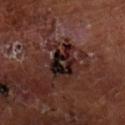The recorded lesion diameter is about 4.5 mm.
This is a cross-polarized tile.
From the arm.
A male patient about 65 years old.
Cropped from a whole-body photographic skin survey; the tile spans about 15 mm.